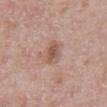Q: Is there a histopathology result?
A: no biopsy performed (imaged during a skin exam)
Q: What is the anatomic site?
A: the abdomen
Q: What kind of image is this?
A: ~15 mm crop, total-body skin-cancer survey
Q: Lesion size?
A: ~3 mm (longest diameter)
Q: What did automated image analysis measure?
A: a lesion area of about 5 mm², a shape eccentricity near 0.75, and a shape-asymmetry score of about 0.2 (0 = symmetric); a lesion–skin lightness drop of about 10 and a lesion-to-skin contrast of about 7 (normalized; higher = more distinct); border irregularity of about 2 on a 0–10 scale; a classifier nevus-likeness of about 35/100 and a lesion-detection confidence of about 100/100
Q: How was the tile lit?
A: white-light
Q: What are the patient's age and sex?
A: male, in their 70s